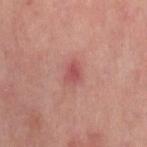follow-up: imaged on a skin check; not biopsied
acquisition: ~15 mm crop, total-body skin-cancer survey
site: the left thigh
tile lighting: cross-polarized
size: ≈2.5 mm
automated metrics: an outline eccentricity of about 0.7 (0 = round, 1 = elongated) and a shape-asymmetry score of about 0.25 (0 = symmetric); a within-lesion color-variation index near 2.5/10 and radial color variation of about 1; a lesion-detection confidence of about 100/100
subject: female, roughly 50 years of age